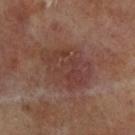The lesion was tiled from a total-body skin photograph and was not biopsied. The lesion's longest dimension is about 6.5 mm. On the leg. Cropped from a whole-body photographic skin survey; the tile spans about 15 mm. A male subject aged around 70. This is a cross-polarized tile.